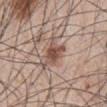A male patient, roughly 45 years of age. Automated image analysis of the tile measured a lesion area of about 8 mm² and two-axis asymmetry of about 0.45. The analysis additionally found a classifier nevus-likeness of about 100/100 and a detector confidence of about 100 out of 100 that the crop contains a lesion. The lesion is located on the abdomen. The recorded lesion diameter is about 4 mm. A 15 mm close-up tile from a total-body photography series done for melanoma screening.